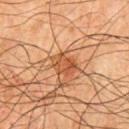Recorded during total-body skin imaging; not selected for excision or biopsy.
A male subject aged approximately 65.
A region of skin cropped from a whole-body photographic capture, roughly 15 mm wide.
On the chest.
This is a cross-polarized tile.
The recorded lesion diameter is about 3.5 mm.
The total-body-photography lesion software estimated an eccentricity of roughly 0.7 and a symmetry-axis asymmetry near 0.2. The software also gave a lesion color around L≈46 a*≈24 b*≈35 in CIELAB, a lesion–skin lightness drop of about 9, and a lesion-to-skin contrast of about 7.5 (normalized; higher = more distinct). It also reported a border-irregularity index near 2/10 and peripheral color asymmetry of about 1.5.